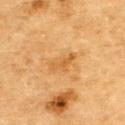biopsy_status: not biopsied; imaged during a skin examination
patient:
  sex: male
  age_approx: 85+
site: upper back
image:
  source: total-body photography crop
  field_of_view_mm: 15